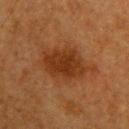| field | value |
|---|---|
| biopsy status | catalogued during a skin exam; not biopsied |
| patient | female, in their mid-50s |
| image source | 15 mm crop, total-body photography |
| lighting | cross-polarized |
| anatomic site | the head or neck |
| automated lesion analysis | an area of roughly 19 mm², an outline eccentricity of about 0.75 (0 = round, 1 = elongated), and two-axis asymmetry of about 0.2; a lesion color around L≈29 a*≈21 b*≈31 in CIELAB and a lesion-to-skin contrast of about 8 (normalized; higher = more distinct); a border-irregularity index near 3/10 and internal color variation of about 2.5 on a 0–10 scale; a nevus-likeness score of about 85/100 |
| size | ~6.5 mm (longest diameter) |